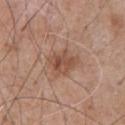Clinical summary: A male subject, in their 60s. A 15 mm crop from a total-body photograph taken for skin-cancer surveillance. About 3.5 mm across. The lesion is on the front of the torso. Automated tile analysis of the lesion measured an automated nevus-likeness rating near 35 out of 100 and lesion-presence confidence of about 100/100.